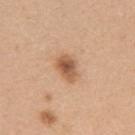automated metrics: a footprint of about 5.5 mm², an outline eccentricity of about 0.7 (0 = round, 1 = elongated), and a shape-asymmetry score of about 0.25 (0 = symmetric); a lesion color around L≈56 a*≈22 b*≈34 in CIELAB, a lesion–skin lightness drop of about 13, and a normalized lesion–skin contrast near 8.5 | subject: female, aged 38 to 42 | tile lighting: white-light | image source: ~15 mm crop, total-body skin-cancer survey | anatomic site: the left upper arm | size: about 3 mm.